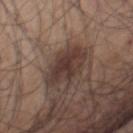workup: no biopsy performed (imaged during a skin exam); body site: the chest; patient: male, aged approximately 55; lesion diameter: ~7 mm (longest diameter); acquisition: ~15 mm tile from a whole-body skin photo.